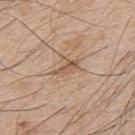Clinical impression: This lesion was catalogued during total-body skin photography and was not selected for biopsy. Context: On the upper back. A close-up tile cropped from a whole-body skin photograph, about 15 mm across. The patient is a male in their mid- to late 40s. The recorded lesion diameter is about 3 mm. Captured under white-light illumination. Automated image analysis of the tile measured a footprint of about 3.5 mm² and an eccentricity of roughly 0.9. And it measured a border-irregularity index near 4/10, internal color variation of about 2.5 on a 0–10 scale, and a peripheral color-asymmetry measure near 0.5. And it measured an automated nevus-likeness rating near 0 out of 100 and a lesion-detection confidence of about 55/100.